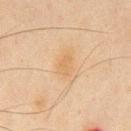{
  "biopsy_status": "not biopsied; imaged during a skin examination",
  "site": "front of the torso",
  "patient": {
    "sex": "male",
    "age_approx": 65
  },
  "automated_metrics": {
    "area_mm2_approx": 4.5,
    "shape_asymmetry": 0.2,
    "cielab_L": 55,
    "cielab_a": 15,
    "cielab_b": 34,
    "vs_skin_darker_L": 5.0,
    "vs_skin_contrast_norm": 5.0,
    "color_variation_0_10": 1.0,
    "peripheral_color_asymmetry": 0.5,
    "nevus_likeness_0_100": 10,
    "lesion_detection_confidence_0_100": 100
  },
  "image": {
    "source": "total-body photography crop",
    "field_of_view_mm": 15
  }
}